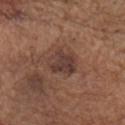biopsy status — catalogued during a skin exam; not biopsied | diameter — about 4 mm | subject — male, aged 78 to 82 | body site — the head or neck | imaging modality — 15 mm crop, total-body photography | tile lighting — white-light.